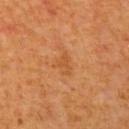The lesion was photographed on a routine skin check and not biopsied; there is no pathology result. The total-body-photography lesion software estimated a lesion area of about 3.5 mm² and two-axis asymmetry of about 0.4. The analysis additionally found roughly 6 lightness units darker than nearby skin. The software also gave a within-lesion color-variation index near 1/10 and peripheral color asymmetry of about 0.5. It also reported a nevus-likeness score of about 0/100. Located on the left upper arm. Imaged with cross-polarized lighting. Approximately 3 mm at its widest. A lesion tile, about 15 mm wide, cut from a 3D total-body photograph. The subject is a female aged approximately 40.